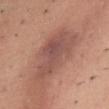Impression: Captured during whole-body skin photography for melanoma surveillance; the lesion was not biopsied. Context: Automated image analysis of the tile measured an area of roughly 25 mm², an eccentricity of roughly 0.85, and a symmetry-axis asymmetry near 0.3. The software also gave a classifier nevus-likeness of about 0/100. Imaged with white-light lighting. The recorded lesion diameter is about 8.5 mm. Located on the chest. The patient is a female aged 38 to 42. A roughly 15 mm field-of-view crop from a total-body skin photograph.